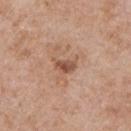Notes:
• workup · catalogued during a skin exam; not biopsied
• image source · ~15 mm tile from a whole-body skin photo
• lesion diameter · about 3 mm
• subject · male, aged 63 to 67
• anatomic site · the front of the torso
• automated metrics · an area of roughly 4 mm², a shape eccentricity near 0.8, and a symmetry-axis asymmetry near 0.3; a border-irregularity index near 3.5/10 and a peripheral color-asymmetry measure near 1; an automated nevus-likeness rating near 30 out of 100 and a detector confidence of about 100 out of 100 that the crop contains a lesion
• illumination · white-light illumination